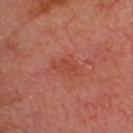No biopsy was performed on this lesion — it was imaged during a full skin examination and was not determined to be concerning.
Measured at roughly 2.5 mm in maximum diameter.
Imaged with cross-polarized lighting.
The subject is a male aged 63 to 67.
A roughly 15 mm field-of-view crop from a total-body skin photograph.
Located on the head or neck.
The lesion-visualizer software estimated an area of roughly 3.5 mm², an outline eccentricity of about 0.8 (0 = round, 1 = elongated), and two-axis asymmetry of about 0.35. And it measured a lesion color around L≈46 a*≈31 b*≈33 in CIELAB, a lesion–skin lightness drop of about 6, and a lesion-to-skin contrast of about 6 (normalized; higher = more distinct). It also reported a nevus-likeness score of about 0/100 and a detector confidence of about 100 out of 100 that the crop contains a lesion.